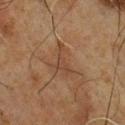follow-up: total-body-photography surveillance lesion; no biopsy
location: the chest
size: ~4 mm (longest diameter)
acquisition: 15 mm crop, total-body photography
illumination: cross-polarized illumination
automated lesion analysis: a lesion area of about 4.5 mm², an outline eccentricity of about 0.85 (0 = round, 1 = elongated), and two-axis asymmetry of about 0.75; border irregularity of about 7.5 on a 0–10 scale, a color-variation rating of about 0.5/10, and a peripheral color-asymmetry measure near 0
subject: male, about 60 years old